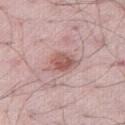notes: catalogued during a skin exam; not biopsied
patient: male, aged approximately 50
location: the right thigh
imaging modality: 15 mm crop, total-body photography
tile lighting: white-light illumination
lesion size: ~4 mm (longest diameter)